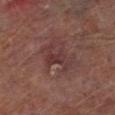No biopsy was performed on this lesion — it was imaged during a full skin examination and was not determined to be concerning.
A male patient, in their mid- to late 60s.
A 15 mm crop from a total-body photograph taken for skin-cancer surveillance.
Automated image analysis of the tile measured border irregularity of about 8 on a 0–10 scale and a within-lesion color-variation index near 3.5/10. The software also gave a classifier nevus-likeness of about 0/100 and a detector confidence of about 90 out of 100 that the crop contains a lesion.
Located on the left lower leg.